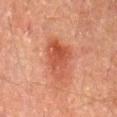follow-up = total-body-photography surveillance lesion; no biopsy
diameter = about 5.5 mm
illumination = cross-polarized illumination
patient = male, aged approximately 60
location = the back
image source = ~15 mm tile from a whole-body skin photo
TBP lesion metrics = an area of roughly 12 mm², an eccentricity of roughly 0.8, and a symmetry-axis asymmetry near 0.35; border irregularity of about 3.5 on a 0–10 scale, internal color variation of about 5 on a 0–10 scale, and radial color variation of about 2; lesion-presence confidence of about 100/100